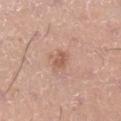The lesion was photographed on a routine skin check and not biopsied; there is no pathology result. The subject is a male aged approximately 35. The tile uses white-light illumination. A roughly 15 mm field-of-view crop from a total-body skin photograph. On the leg. The total-body-photography lesion software estimated a lesion area of about 3.5 mm², an eccentricity of roughly 0.8, and two-axis asymmetry of about 0.25. The analysis additionally found border irregularity of about 2.5 on a 0–10 scale, internal color variation of about 1.5 on a 0–10 scale, and peripheral color asymmetry of about 0.5. Measured at roughly 2.5 mm in maximum diameter.